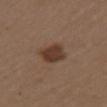Assessment:
No biopsy was performed on this lesion — it was imaged during a full skin examination and was not determined to be concerning.
Background:
The patient is a female in their 40s. A region of skin cropped from a whole-body photographic capture, roughly 15 mm wide. The lesion is located on the arm. Automated image analysis of the tile measured border irregularity of about 1.5 on a 0–10 scale and a color-variation rating of about 2.5/10. The analysis additionally found a classifier nevus-likeness of about 90/100 and lesion-presence confidence of about 100/100. This is a white-light tile. Approximately 3.5 mm at its widest.